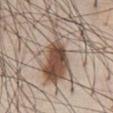biopsy status: imaged on a skin check; not biopsied
lesion diameter: ~10.5 mm (longest diameter)
automated metrics: a classifier nevus-likeness of about 65/100 and lesion-presence confidence of about 55/100
location: the chest
illumination: white-light
subject: male, roughly 60 years of age
imaging modality: ~15 mm tile from a whole-body skin photo